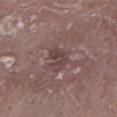Impression: The lesion was tiled from a total-body skin photograph and was not biopsied. Image and clinical context: A male patient, aged around 80. An algorithmic analysis of the crop reported an area of roughly 5 mm², a shape eccentricity near 0.7, and a shape-asymmetry score of about 0.3 (0 = symmetric). And it measured a mean CIELAB color near L≈41 a*≈17 b*≈16 and roughly 8 lightness units darker than nearby skin. The analysis additionally found an automated nevus-likeness rating near 0 out of 100. The tile uses white-light illumination. The lesion is located on the right lower leg. A roughly 15 mm field-of-view crop from a total-body skin photograph.